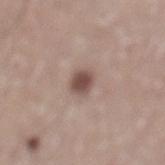  biopsy_status: not biopsied; imaged during a skin examination
  image:
    source: total-body photography crop
    field_of_view_mm: 15
  site: mid back
  lesion_size:
    long_diameter_mm_approx: 2.5
  patient:
    sex: male
    age_approx: 75
  lighting: white-light